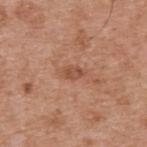automated_metrics:
  area_mm2_approx: 3.5
  shape_asymmetry: 0.35
  cielab_L: 51
  cielab_a: 24
  cielab_b: 32
  vs_skin_contrast_norm: 6.0
  border_irregularity_0_10: 3.0
  color_variation_0_10: 1.5
  peripheral_color_asymmetry: 0.5
site: upper back
image:
  source: total-body photography crop
  field_of_view_mm: 15
patient:
  sex: male
  age_approx: 55
lighting: white-light
lesion_size:
  long_diameter_mm_approx: 3.0